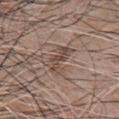Notes:
- biopsy status · imaged on a skin check; not biopsied
- lesion diameter · ≈4 mm
- patient · male, about 75 years old
- image · ~15 mm crop, total-body skin-cancer survey
- automated metrics · an outline eccentricity of about 0.9 (0 = round, 1 = elongated) and a shape-asymmetry score of about 0.45 (0 = symmetric)
- tile lighting · white-light
- anatomic site · the chest